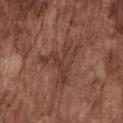Impression: The lesion was photographed on a routine skin check and not biopsied; there is no pathology result. Image and clinical context: A roughly 15 mm field-of-view crop from a total-body skin photograph. The lesion's longest dimension is about 6 mm. On the chest. The total-body-photography lesion software estimated an eccentricity of roughly 0.6 and a shape-asymmetry score of about 0.7 (0 = symmetric). It also reported a normalized border contrast of about 6. And it measured a nevus-likeness score of about 0/100. The tile uses white-light illumination. A male patient aged 73–77.